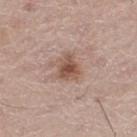lesion size = ≈3 mm; subject = male, roughly 70 years of age; acquisition = ~15 mm crop, total-body skin-cancer survey; illumination = white-light illumination; anatomic site = the right leg.